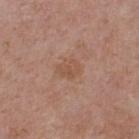Assessment:
Part of a total-body skin-imaging series; this lesion was reviewed on a skin check and was not flagged for biopsy.
Context:
A roughly 15 mm field-of-view crop from a total-body skin photograph. Located on the upper back. A female subject in their 60s.